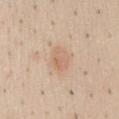Findings:
– follow-up · catalogued during a skin exam; not biopsied
– illumination · white-light
– size · ~3 mm (longest diameter)
– acquisition · ~15 mm tile from a whole-body skin photo
– subject · male, aged approximately 35
– location · the abdomen